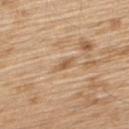Clinical impression: Captured during whole-body skin photography for melanoma surveillance; the lesion was not biopsied. Context: A 15 mm close-up extracted from a 3D total-body photography capture. The total-body-photography lesion software estimated a lesion area of about 2.5 mm², a shape eccentricity near 0.9, and a shape-asymmetry score of about 0.35 (0 = symmetric). It also reported a lesion color around L≈58 a*≈18 b*≈35 in CIELAB and a normalized lesion–skin contrast near 6.5. It also reported border irregularity of about 3.5 on a 0–10 scale. About 2.5 mm across. This is a white-light tile. The lesion is located on the upper back. The subject is a male roughly 70 years of age.